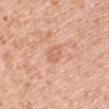| key | value |
|---|---|
| follow-up | total-body-photography surveillance lesion; no biopsy |
| size | ≈3 mm |
| illumination | white-light illumination |
| patient | male, in their mid-40s |
| body site | the right upper arm |
| image-analysis metrics | a mean CIELAB color near L≈65 a*≈24 b*≈34, about 7 CIELAB-L* units darker than the surrounding skin, and a normalized lesion–skin contrast near 5; a border-irregularity rating of about 2.5/10 and peripheral color asymmetry of about 1 |
| acquisition | total-body-photography crop, ~15 mm field of view |